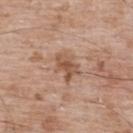anatomic site = the upper back | illumination = white-light | diameter = ≈3.5 mm | subject = male, aged around 50 | acquisition = ~15 mm tile from a whole-body skin photo.